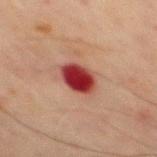Captured during whole-body skin photography for melanoma surveillance; the lesion was not biopsied. The lesion is located on the chest. An algorithmic analysis of the crop reported a lesion–skin lightness drop of about 17 and a normalized border contrast of about 14.5. And it measured a color-variation rating of about 4.5/10 and a peripheral color-asymmetry measure near 1.5. And it measured an automated nevus-likeness rating near 0 out of 100 and lesion-presence confidence of about 100/100. A 15 mm crop from a total-body photograph taken for skin-cancer surveillance. A male patient aged approximately 70. Measured at roughly 3.5 mm in maximum diameter.